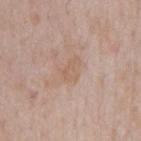The lesion was tiled from a total-body skin photograph and was not biopsied.
A lesion tile, about 15 mm wide, cut from a 3D total-body photograph.
Automated tile analysis of the lesion measured an area of roughly 4.5 mm² and two-axis asymmetry of about 0.45. And it measured an average lesion color of about L≈60 a*≈17 b*≈29 (CIELAB) and a normalized border contrast of about 5. It also reported border irregularity of about 5 on a 0–10 scale, internal color variation of about 2 on a 0–10 scale, and peripheral color asymmetry of about 0.5.
Longest diameter approximately 3 mm.
The lesion is on the front of the torso.
Captured under white-light illumination.
A male patient aged around 65.